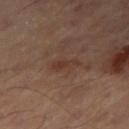{
  "biopsy_status": "not biopsied; imaged during a skin examination",
  "image": {
    "source": "total-body photography crop",
    "field_of_view_mm": 15
  },
  "site": "leg"
}